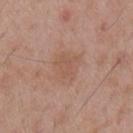{"biopsy_status": "not biopsied; imaged during a skin examination", "lesion_size": {"long_diameter_mm_approx": 3.5}, "automated_metrics": {"shape_asymmetry": 0.2, "cielab_L": 55, "cielab_a": 20, "cielab_b": 28, "vs_skin_darker_L": 6.0, "vs_skin_contrast_norm": 4.5, "nevus_likeness_0_100": 0, "lesion_detection_confidence_0_100": 100}, "lighting": "white-light", "patient": {"sex": "male", "age_approx": 55}, "site": "mid back", "image": {"source": "total-body photography crop", "field_of_view_mm": 15}}